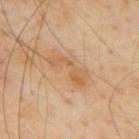The lesion was tiled from a total-body skin photograph and was not biopsied.
Imaged with cross-polarized lighting.
A 15 mm crop from a total-body photograph taken for skin-cancer surveillance.
A male patient, aged 53–57.
The total-body-photography lesion software estimated a border-irregularity rating of about 7/10 and a color-variation rating of about 3/10.
Located on the back.
Measured at roughly 5 mm in maximum diameter.